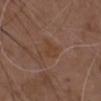Assessment:
No biopsy was performed on this lesion — it was imaged during a full skin examination and was not determined to be concerning.
Image and clinical context:
The tile uses white-light illumination. A male subject, aged around 75. The lesion is located on the chest. Cropped from a total-body skin-imaging series; the visible field is about 15 mm. The recorded lesion diameter is about 3 mm.